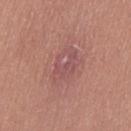Q: Was a biopsy performed?
A: no biopsy performed (imaged during a skin exam)
Q: What kind of image is this?
A: total-body-photography crop, ~15 mm field of view
Q: Lesion location?
A: the left thigh
Q: Who is the patient?
A: female, in their mid-20s
Q: Illumination type?
A: white-light illumination